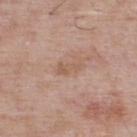Captured during whole-body skin photography for melanoma surveillance; the lesion was not biopsied.
A male patient, in their mid- to late 60s.
The total-body-photography lesion software estimated a lesion area of about 3 mm² and an outline eccentricity of about 0.9 (0 = round, 1 = elongated). And it measured a lesion–skin lightness drop of about 7 and a normalized border contrast of about 5.5. It also reported a within-lesion color-variation index near 0/10 and peripheral color asymmetry of about 0. The software also gave an automated nevus-likeness rating near 0 out of 100 and lesion-presence confidence of about 100/100.
A 15 mm close-up tile from a total-body photography series done for melanoma screening.
The lesion's longest dimension is about 3 mm.
The lesion is located on the upper back.